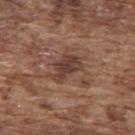Measured at roughly 4 mm in maximum diameter.
Imaged with white-light lighting.
A male subject aged around 75.
The lesion-visualizer software estimated an automated nevus-likeness rating near 0 out of 100 and lesion-presence confidence of about 100/100.
On the upper back.
A 15 mm close-up extracted from a 3D total-body photography capture.